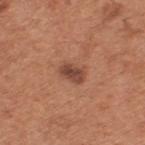Imaged during a routine full-body skin examination; the lesion was not biopsied and no histopathology is available. A 15 mm crop from a total-body photograph taken for skin-cancer surveillance. The subject is a male aged 63–67. About 3 mm across. Imaged with white-light lighting. On the back.